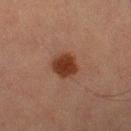Part of a total-body skin-imaging series; this lesion was reviewed on a skin check and was not flagged for biopsy. A roughly 15 mm field-of-view crop from a total-body skin photograph. Approximately 3 mm at its widest. The total-body-photography lesion software estimated a lesion area of about 7 mm², an eccentricity of roughly 0.5, and two-axis asymmetry of about 0.15. Imaged with cross-polarized lighting. The lesion is on the right lower leg. The patient is a female roughly 60 years of age.